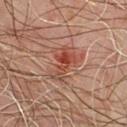Q: Was this lesion biopsied?
A: catalogued during a skin exam; not biopsied
Q: Where on the body is the lesion?
A: the chest
Q: What kind of image is this?
A: 15 mm crop, total-body photography
Q: Illumination type?
A: cross-polarized
Q: What are the patient's age and sex?
A: male, aged 43–47
Q: What did automated image analysis measure?
A: a footprint of about 8.5 mm², an eccentricity of roughly 0.8, and two-axis asymmetry of about 0.5; an average lesion color of about L≈38 a*≈22 b*≈25 (CIELAB) and a lesion-to-skin contrast of about 7.5 (normalized; higher = more distinct); a border-irregularity index near 6/10 and a peripheral color-asymmetry measure near 2; a nevus-likeness score of about 10/100 and lesion-presence confidence of about 100/100